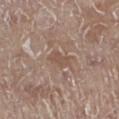Assessment:
Part of a total-body skin-imaging series; this lesion was reviewed on a skin check and was not flagged for biopsy.
Image and clinical context:
Imaged with white-light lighting. A male patient, approximately 70 years of age. Approximately 3 mm at its widest. The lesion is on the leg. A 15 mm close-up tile from a total-body photography series done for melanoma screening.